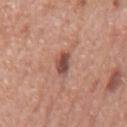Captured during whole-body skin photography for melanoma surveillance; the lesion was not biopsied. A region of skin cropped from a whole-body photographic capture, roughly 15 mm wide. The lesion is on the mid back. Approximately 2.5 mm at its widest. The subject is a male approximately 75 years of age. Imaged with white-light lighting.